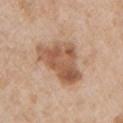Captured during whole-body skin photography for melanoma surveillance; the lesion was not biopsied.
Automated image analysis of the tile measured an eccentricity of roughly 0.75. The software also gave a border-irregularity index near 4.5/10, internal color variation of about 5.5 on a 0–10 scale, and peripheral color asymmetry of about 2. And it measured an automated nevus-likeness rating near 5 out of 100 and a lesion-detection confidence of about 100/100.
Cropped from a whole-body photographic skin survey; the tile spans about 15 mm.
The subject is a male in their mid-60s.
This is a white-light tile.
Approximately 7 mm at its widest.
From the arm.